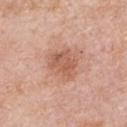Notes:
* workup · total-body-photography surveillance lesion; no biopsy
* image source · total-body-photography crop, ~15 mm field of view
* location · the right upper arm
* subject · male, aged 73–77